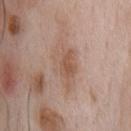Captured during whole-body skin photography for melanoma surveillance; the lesion was not biopsied.
From the chest.
A 15 mm crop from a total-body photograph taken for skin-cancer surveillance.
The subject is a male roughly 60 years of age.